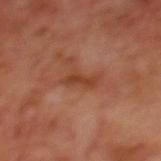Imaged during a routine full-body skin examination; the lesion was not biopsied and no histopathology is available.
On the mid back.
A male subject, roughly 70 years of age.
Cropped from a total-body skin-imaging series; the visible field is about 15 mm.
The tile uses cross-polarized illumination.
The lesion's longest dimension is about 3.5 mm.